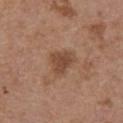* notes — catalogued during a skin exam; not biopsied
* imaging modality — 15 mm crop, total-body photography
* location — the abdomen
* lighting — white-light
* subject — female, aged approximately 65
* size — ≈3 mm
* automated metrics — a lesion color around L≈45 a*≈20 b*≈30 in CIELAB, a lesion–skin lightness drop of about 9, and a lesion-to-skin contrast of about 7.5 (normalized; higher = more distinct)